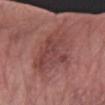Impression:
Imaged during a routine full-body skin examination; the lesion was not biopsied and no histopathology is available.
Image and clinical context:
Approximately 7 mm at its widest. Cropped from a total-body skin-imaging series; the visible field is about 15 mm. This is a white-light tile. A female subject aged 83 or over. From the right forearm. An algorithmic analysis of the crop reported an area of roughly 22 mm², an eccentricity of roughly 0.75, and two-axis asymmetry of about 0.3. The analysis additionally found a mean CIELAB color near L≈44 a*≈24 b*≈22, roughly 8 lightness units darker than nearby skin, and a normalized lesion–skin contrast near 6. It also reported an automated nevus-likeness rating near 0 out of 100.